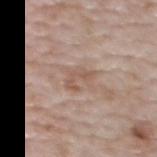Findings:
- location · the back
- patient · female, about 65 years old
- lesion diameter · ~3.5 mm (longest diameter)
- image source · ~15 mm tile from a whole-body skin photo
- tile lighting · white-light illumination